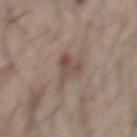Background:
Measured at roughly 4 mm in maximum diameter. A 15 mm close-up tile from a total-body photography series done for melanoma screening. The lesion is located on the front of the torso. The subject is a male aged approximately 75.